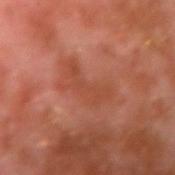The lesion was tiled from a total-body skin photograph and was not biopsied.